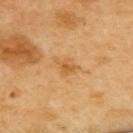<case>
<biopsy_status>not biopsied; imaged during a skin examination</biopsy_status>
<site>upper back</site>
<patient>
  <sex>female</sex>
  <age_approx>55</age_approx>
</patient>
<image>
  <source>total-body photography crop</source>
  <field_of_view_mm>15</field_of_view_mm>
</image>
</case>